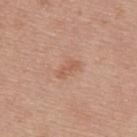Notes:
– follow-up — catalogued during a skin exam; not biopsied
– subject — female, about 65 years old
– lighting — white-light illumination
– size — ~3 mm (longest diameter)
– imaging modality — ~15 mm tile from a whole-body skin photo
– site — the upper back